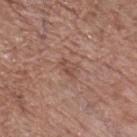Recorded during total-body skin imaging; not selected for excision or biopsy. Located on the back. This is a white-light tile. The lesion's longest dimension is about 2.5 mm. A close-up tile cropped from a whole-body skin photograph, about 15 mm across. The total-body-photography lesion software estimated a mean CIELAB color near L≈49 a*≈21 b*≈26, a lesion–skin lightness drop of about 7, and a normalized border contrast of about 5.5. The analysis additionally found border irregularity of about 3.5 on a 0–10 scale, internal color variation of about 2 on a 0–10 scale, and a peripheral color-asymmetry measure near 0.5. A male patient aged 68–72.